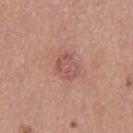{
  "biopsy_status": "not biopsied; imaged during a skin examination",
  "site": "left thigh",
  "patient": {
    "sex": "female",
    "age_approx": 35
  },
  "image": {
    "source": "total-body photography crop",
    "field_of_view_mm": 15
  },
  "lesion_size": {
    "long_diameter_mm_approx": 3.0
  },
  "lighting": "white-light",
  "automated_metrics": {
    "eccentricity": 0.6,
    "cielab_L": 54,
    "cielab_a": 25,
    "cielab_b": 25,
    "vs_skin_darker_L": 8.0,
    "vs_skin_contrast_norm": 5.5,
    "border_irregularity_0_10": 3.0,
    "peripheral_color_asymmetry": 1.0
  }
}